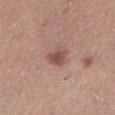Image and clinical context:
A female patient aged 28 to 32. Imaged with white-light lighting. A region of skin cropped from a whole-body photographic capture, roughly 15 mm wide. Approximately 2.5 mm at its widest. From the left lower leg.